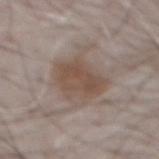Assessment: Imaged during a routine full-body skin examination; the lesion was not biopsied and no histopathology is available. Image and clinical context: A 15 mm crop from a total-body photograph taken for skin-cancer surveillance. From the abdomen. The subject is a male aged around 70. The total-body-photography lesion software estimated a shape eccentricity near 0.6 and two-axis asymmetry of about 0.25. And it measured roughly 9 lightness units darker than nearby skin and a lesion-to-skin contrast of about 7.5 (normalized; higher = more distinct). It also reported a border-irregularity index near 4/10, internal color variation of about 4 on a 0–10 scale, and a peripheral color-asymmetry measure near 1. And it measured lesion-presence confidence of about 100/100.